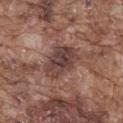biopsy status: catalogued during a skin exam; not biopsied
site: the mid back
image-analysis metrics: an area of roughly 14 mm², an outline eccentricity of about 0.7 (0 = round, 1 = elongated), and a symmetry-axis asymmetry near 0.25; a border-irregularity rating of about 3/10 and a color-variation rating of about 5/10; a classifier nevus-likeness of about 0/100 and a detector confidence of about 95 out of 100 that the crop contains a lesion
size: ~5 mm (longest diameter)
subject: male, aged around 75
image: ~15 mm tile from a whole-body skin photo
tile lighting: white-light illumination